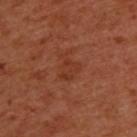{
  "biopsy_status": "not biopsied; imaged during a skin examination",
  "image": {
    "source": "total-body photography crop",
    "field_of_view_mm": 15
  },
  "lesion_size": {
    "long_diameter_mm_approx": 3.5
  },
  "automated_metrics": {
    "area_mm2_approx": 5.0,
    "eccentricity": 0.7,
    "border_irregularity_0_10": 5.5,
    "peripheral_color_asymmetry": 0.5
  },
  "patient": {
    "sex": "male",
    "age_approx": 50
  },
  "site": "upper back"
}